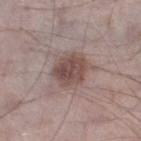follow-up: total-body-photography surveillance lesion; no biopsy | body site: the left lower leg | acquisition: ~15 mm crop, total-body skin-cancer survey | patient: male, about 70 years old.